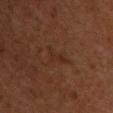notes = no biopsy performed (imaged during a skin exam) | image = ~15 mm tile from a whole-body skin photo | tile lighting = cross-polarized | body site = the chest | image-analysis metrics = a lesion area of about 2.5 mm² and a shape eccentricity near 0.9; a color-variation rating of about 0/10 and a peripheral color-asymmetry measure near 0; a nevus-likeness score of about 0/100 and lesion-presence confidence of about 100/100 | lesion diameter = ≈3 mm | patient = female, aged 48–52.